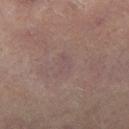Q: Is there a histopathology result?
A: imaged on a skin check; not biopsied
Q: Where on the body is the lesion?
A: the right thigh
Q: What are the patient's age and sex?
A: male, about 55 years old
Q: How large is the lesion?
A: ≈2.5 mm
Q: What kind of image is this?
A: total-body-photography crop, ~15 mm field of view
Q: What lighting was used for the tile?
A: cross-polarized illumination